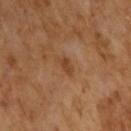workup=no biopsy performed (imaged during a skin exam) | illumination=cross-polarized | acquisition=total-body-photography crop, ~15 mm field of view | patient=male, aged approximately 65 | size=~2.5 mm (longest diameter).